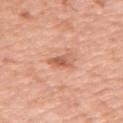Assessment: Part of a total-body skin-imaging series; this lesion was reviewed on a skin check and was not flagged for biopsy. Clinical summary: A female subject about 55 years old. Captured under white-light illumination. The lesion is located on the arm. About 3 mm across. A 15 mm close-up tile from a total-body photography series done for melanoma screening. The lesion-visualizer software estimated an area of roughly 4.5 mm², an outline eccentricity of about 0.8 (0 = round, 1 = elongated), and a shape-asymmetry score of about 0.4 (0 = symmetric).